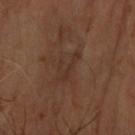notes = imaged on a skin check; not biopsied | imaging modality = 15 mm crop, total-body photography | location = the right forearm | automated lesion analysis = a footprint of about 4 mm² and an eccentricity of roughly 0.9; a border-irregularity rating of about 9/10, a color-variation rating of about 0.5/10, and peripheral color asymmetry of about 0 | patient = in their mid- to late 60s.